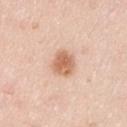- workup — total-body-photography surveillance lesion; no biopsy
- size — ~3 mm (longest diameter)
- subject — male, aged approximately 35
- imaging modality — ~15 mm tile from a whole-body skin photo
- lighting — white-light illumination
- location — the left upper arm
- TBP lesion metrics — a lesion area of about 6.5 mm² and a symmetry-axis asymmetry near 0.2; a border-irregularity rating of about 2/10 and a color-variation rating of about 3/10; a lesion-detection confidence of about 100/100